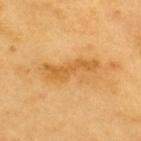Impression: Recorded during total-body skin imaging; not selected for excision or biopsy. Acquisition and patient details: About 6.5 mm across. Located on the upper back. The tile uses cross-polarized illumination. A male patient aged 58 to 62. An algorithmic analysis of the crop reported a lesion area of about 12 mm², an outline eccentricity of about 0.95 (0 = round, 1 = elongated), and a shape-asymmetry score of about 0.35 (0 = symmetric). The software also gave a mean CIELAB color near L≈61 a*≈22 b*≈48 and a normalized lesion–skin contrast near 6.5. It also reported a border-irregularity rating of about 6/10 and a within-lesion color-variation index near 3/10. A roughly 15 mm field-of-view crop from a total-body skin photograph.